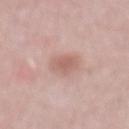Recorded during total-body skin imaging; not selected for excision or biopsy.
The lesion is on the abdomen.
A region of skin cropped from a whole-body photographic capture, roughly 15 mm wide.
Approximately 3.5 mm at its widest.
The tile uses white-light illumination.
A male subject about 50 years old.